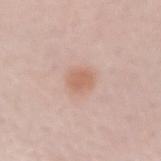Impression: No biopsy was performed on this lesion — it was imaged during a full skin examination and was not determined to be concerning. Clinical summary: The recorded lesion diameter is about 2.5 mm. The lesion-visualizer software estimated an area of roughly 4.5 mm², an outline eccentricity of about 0.6 (0 = round, 1 = elongated), and a shape-asymmetry score of about 0.2 (0 = symmetric). It also reported a mean CIELAB color near L≈62 a*≈21 b*≈29, roughly 8 lightness units darker than nearby skin, and a lesion-to-skin contrast of about 6.5 (normalized; higher = more distinct). The software also gave a nevus-likeness score of about 95/100 and lesion-presence confidence of about 100/100. A lesion tile, about 15 mm wide, cut from a 3D total-body photograph. Located on the left forearm. The subject is a female in their mid- to late 40s. Imaged with white-light lighting.